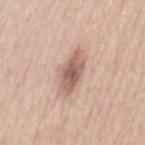- follow-up: no biopsy performed (imaged during a skin exam)
- patient: male, aged approximately 45
- anatomic site: the lower back
- lesion size: ≈6 mm
- tile lighting: white-light illumination
- image source: ~15 mm tile from a whole-body skin photo
- automated metrics: a lesion color around L≈59 a*≈20 b*≈24 in CIELAB, roughly 14 lightness units darker than nearby skin, and a normalized lesion–skin contrast near 9